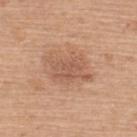| key | value |
|---|---|
| subject | female, about 60 years old |
| acquisition | ~15 mm tile from a whole-body skin photo |
| anatomic site | the back |
| lesion size | about 5 mm |